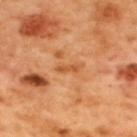biopsy status — no biopsy performed (imaged during a skin exam)
subject — male, approximately 50 years of age
acquisition — 15 mm crop, total-body photography
anatomic site — the back